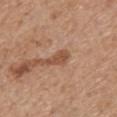Q: Was this lesion biopsied?
A: total-body-photography surveillance lesion; no biopsy
Q: What are the patient's age and sex?
A: male, aged 68–72
Q: What lighting was used for the tile?
A: white-light
Q: How large is the lesion?
A: ~3 mm (longest diameter)
Q: How was this image acquired?
A: ~15 mm crop, total-body skin-cancer survey
Q: What did automated image analysis measure?
A: a nevus-likeness score of about 5/100 and a detector confidence of about 100 out of 100 that the crop contains a lesion
Q: What is the anatomic site?
A: the back